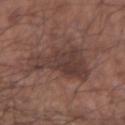Notes:
- biopsy status: catalogued during a skin exam; not biopsied
- patient: male, in their mid- to late 50s
- acquisition: ~15 mm crop, total-body skin-cancer survey
- body site: the left forearm